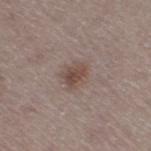workup = imaged on a skin check; not biopsied | body site = the right thigh | patient = male, aged 63 to 67 | acquisition = ~15 mm crop, total-body skin-cancer survey | lighting = white-light | lesion diameter = ≈3 mm.